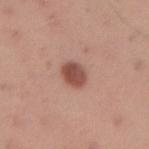Clinical impression:
Imaged during a routine full-body skin examination; the lesion was not biopsied and no histopathology is available.
Background:
Measured at roughly 3 mm in maximum diameter. Automated tile analysis of the lesion measured a lesion area of about 6 mm², a shape eccentricity near 0.5, and a shape-asymmetry score of about 0.1 (0 = symmetric). The analysis additionally found a border-irregularity rating of about 1/10, a within-lesion color-variation index near 3/10, and radial color variation of about 1. The software also gave a classifier nevus-likeness of about 100/100 and a lesion-detection confidence of about 100/100. Cropped from a whole-body photographic skin survey; the tile spans about 15 mm. From the right upper arm. Captured under white-light illumination. A male patient, aged approximately 35.